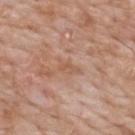Impression:
Part of a total-body skin-imaging series; this lesion was reviewed on a skin check and was not flagged for biopsy.
Acquisition and patient details:
The lesion is located on the mid back. The recorded lesion diameter is about 2.5 mm. A male patient, roughly 60 years of age. This is a white-light tile. A roughly 15 mm field-of-view crop from a total-body skin photograph.